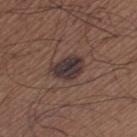Impression: Imaged during a routine full-body skin examination; the lesion was not biopsied and no histopathology is available. Clinical summary: The tile uses white-light illumination. Automated image analysis of the tile measured a footprint of about 8 mm², a shape eccentricity near 0.75, and a symmetry-axis asymmetry near 0.25. The software also gave a lesion color around L≈32 a*≈13 b*≈15 in CIELAB, roughly 11 lightness units darker than nearby skin, and a lesion-to-skin contrast of about 12.5 (normalized; higher = more distinct). The analysis additionally found a border-irregularity index near 2.5/10, internal color variation of about 4 on a 0–10 scale, and peripheral color asymmetry of about 1.5. The lesion is located on the left thigh. A region of skin cropped from a whole-body photographic capture, roughly 15 mm wide. The lesion's longest dimension is about 4 mm. A male subject aged around 65.